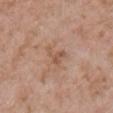Clinical impression: The lesion was photographed on a routine skin check and not biopsied; there is no pathology result. Background: A region of skin cropped from a whole-body photographic capture, roughly 15 mm wide. A male subject approximately 50 years of age. The recorded lesion diameter is about 3 mm. Automated tile analysis of the lesion measured a lesion color around L≈54 a*≈19 b*≈30 in CIELAB and a lesion–skin lightness drop of about 7. The analysis additionally found a border-irregularity index near 6.5/10 and a color-variation rating of about 1.5/10. Located on the chest.